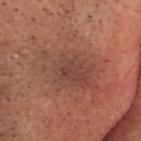Imaged during a routine full-body skin examination; the lesion was not biopsied and no histopathology is available.
Imaged with cross-polarized lighting.
The patient is a male roughly 55 years of age.
The lesion-visualizer software estimated a footprint of about 24 mm², a shape eccentricity near 0.85, and a shape-asymmetry score of about 0.25 (0 = symmetric). And it measured a lesion–skin lightness drop of about 6 and a normalized lesion–skin contrast near 6. It also reported a nevus-likeness score of about 0/100 and lesion-presence confidence of about 95/100.
The lesion is located on the head or neck.
Cropped from a whole-body photographic skin survey; the tile spans about 15 mm.
Longest diameter approximately 8 mm.